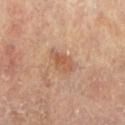<record>
<biopsy_status>not biopsied; imaged during a skin examination</biopsy_status>
<lesion_size>
  <long_diameter_mm_approx>3.5</long_diameter_mm_approx>
</lesion_size>
<patient>
  <sex>female</sex>
  <age_approx>75</age_approx>
</patient>
<automated_metrics>
  <eccentricity>0.85</eccentricity>
  <shape_asymmetry>0.3</shape_asymmetry>
</automated_metrics>
<image>
  <source>total-body photography crop</source>
  <field_of_view_mm>15</field_of_view_mm>
</image>
<site>right lower leg</site>
</record>